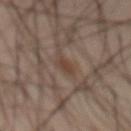Clinical impression:
Part of a total-body skin-imaging series; this lesion was reviewed on a skin check and was not flagged for biopsy.
Context:
This is a cross-polarized tile. Automated tile analysis of the lesion measured an area of roughly 4 mm² and an eccentricity of roughly 0.85. It also reported a border-irregularity index near 3/10. The analysis additionally found a detector confidence of about 95 out of 100 that the crop contains a lesion. The lesion is located on the abdomen. A male subject about 45 years old. A 15 mm close-up tile from a total-body photography series done for melanoma screening.